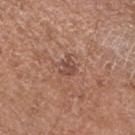Notes:
– follow-up: imaged on a skin check; not biopsied
– site: the right upper arm
– patient: male, roughly 70 years of age
– image source: ~15 mm tile from a whole-body skin photo